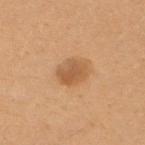Clinical impression:
Imaged during a routine full-body skin examination; the lesion was not biopsied and no histopathology is available.
Acquisition and patient details:
A female patient, roughly 30 years of age. A 15 mm close-up tile from a total-body photography series done for melanoma screening. The lesion is located on the upper back.